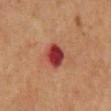follow-up = no biopsy performed (imaged during a skin exam) | subject = male, in their 60s | lighting = cross-polarized | site = the mid back | image = ~15 mm crop, total-body skin-cancer survey | diameter = ~3 mm (longest diameter) | image-analysis metrics = lesion-presence confidence of about 100/100.